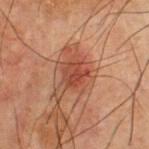Case summary:
* workup: imaged on a skin check; not biopsied
* image source: total-body-photography crop, ~15 mm field of view
* site: the chest
* tile lighting: cross-polarized
* patient: male, approximately 70 years of age
* diameter: ~2.5 mm (longest diameter)